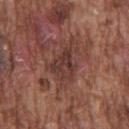* follow-up — imaged on a skin check; not biopsied
* body site — the front of the torso
* acquisition — 15 mm crop, total-body photography
* lesion size — ~4 mm (longest diameter)
* subject — male, in their mid- to late 70s
* automated lesion analysis — an area of roughly 7.5 mm², an outline eccentricity of about 0.7 (0 = round, 1 = elongated), and a symmetry-axis asymmetry near 0.5; a lesion color around L≈36 a*≈21 b*≈22 in CIELAB, a lesion–skin lightness drop of about 8, and a normalized border contrast of about 7; a nevus-likeness score of about 0/100